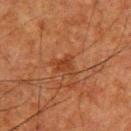workup=no biopsy performed (imaged during a skin exam) | diameter=~2.5 mm (longest diameter) | image=15 mm crop, total-body photography | subject=male, aged around 65 | automated lesion analysis=an average lesion color of about L≈33 a*≈23 b*≈31 (CIELAB), a lesion–skin lightness drop of about 6, and a normalized lesion–skin contrast near 6; a border-irregularity index near 4.5/10 and a within-lesion color-variation index near 1.5/10 | anatomic site=the upper back.